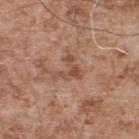Recorded during total-body skin imaging; not selected for excision or biopsy. A male patient, aged around 55. Automated image analysis of the tile measured an outline eccentricity of about 0.5 (0 = round, 1 = elongated). From the upper back. A region of skin cropped from a whole-body photographic capture, roughly 15 mm wide.